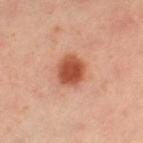site: the right thigh; image: total-body-photography crop, ~15 mm field of view; lighting: cross-polarized; lesion size: ~3.5 mm (longest diameter); subject: female, aged around 40.